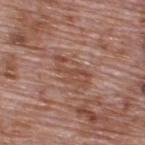Assessment: Captured during whole-body skin photography for melanoma surveillance; the lesion was not biopsied. Acquisition and patient details: This is a white-light tile. About 5 mm across. A male patient, aged around 70. The lesion is on the upper back. Cropped from a total-body skin-imaging series; the visible field is about 15 mm.